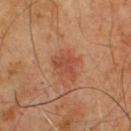Imaged during a routine full-body skin examination; the lesion was not biopsied and no histopathology is available.
An algorithmic analysis of the crop reported a footprint of about 6.5 mm² and two-axis asymmetry of about 0.4. It also reported a mean CIELAB color near L≈47 a*≈27 b*≈32, roughly 8 lightness units darker than nearby skin, and a normalized lesion–skin contrast near 6. The software also gave a nevus-likeness score of about 55/100 and lesion-presence confidence of about 100/100.
Approximately 3.5 mm at its widest.
A male patient aged approximately 65.
The tile uses cross-polarized illumination.
This image is a 15 mm lesion crop taken from a total-body photograph.